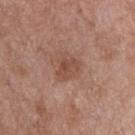Part of a total-body skin-imaging series; this lesion was reviewed on a skin check and was not flagged for biopsy. This is a white-light tile. Cropped from a total-body skin-imaging series; the visible field is about 15 mm. From the chest. About 3 mm across. The subject is a male in their 50s.